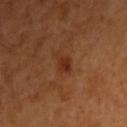Findings:
• notes: catalogued during a skin exam; not biopsied
• subject: male, aged around 50
• image: ~15 mm tile from a whole-body skin photo
• location: the right upper arm